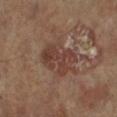Part of a total-body skin-imaging series; this lesion was reviewed on a skin check and was not flagged for biopsy. Longest diameter approximately 4.5 mm. A female patient aged 73 to 77. A lesion tile, about 15 mm wide, cut from a 3D total-body photograph. On the right lower leg. The total-body-photography lesion software estimated an area of roughly 13 mm² and a shape eccentricity near 0.7. It also reported an average lesion color of about L≈39 a*≈19 b*≈24 (CIELAB) and a normalized lesion–skin contrast near 7. It also reported a border-irregularity rating of about 4/10, a color-variation rating of about 4/10, and a peripheral color-asymmetry measure near 1.5. It also reported a classifier nevus-likeness of about 0/100 and a detector confidence of about 100 out of 100 that the crop contains a lesion.